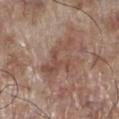lesion_size:
  long_diameter_mm_approx: 5.5
patient:
  sex: male
  age_approx: 70
site: leg
automated_metrics:
  area_mm2_approx: 13.0
  eccentricity: 0.75
  shape_asymmetry: 0.45
  lesion_detection_confidence_0_100: 90
image:
  source: total-body photography crop
  field_of_view_mm: 15
lighting: white-light